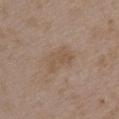Case summary:
• biopsy status · imaged on a skin check; not biopsied
• patient · female, aged approximately 35
• site · the chest
• automated lesion analysis · an area of roughly 7.5 mm², a shape eccentricity near 0.8, and a shape-asymmetry score of about 0.3 (0 = symmetric); a mean CIELAB color near L≈53 a*≈15 b*≈28 and a lesion–skin lightness drop of about 6; a classifier nevus-likeness of about 0/100 and a detector confidence of about 100 out of 100 that the crop contains a lesion
• imaging modality · ~15 mm tile from a whole-body skin photo